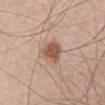The lesion was tiled from a total-body skin photograph and was not biopsied.
Imaged with white-light lighting.
A lesion tile, about 15 mm wide, cut from a 3D total-body photograph.
A male subject, roughly 35 years of age.
The lesion is on the back.